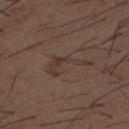Part of a total-body skin-imaging series; this lesion was reviewed on a skin check and was not flagged for biopsy. The total-body-photography lesion software estimated a lesion color around L≈35 a*≈13 b*≈22 in CIELAB. The software also gave a lesion-detection confidence of about 90/100. A 15 mm close-up extracted from a 3D total-body photography capture. The subject is a male aged approximately 50. The recorded lesion diameter is about 5 mm. The lesion is on the back. Imaged with white-light lighting.